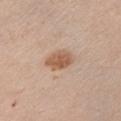No biopsy was performed on this lesion — it was imaged during a full skin examination and was not determined to be concerning.